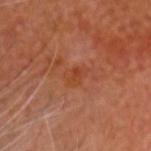follow-up = no biopsy performed (imaged during a skin exam); location = the head or neck; patient = male, in their mid-60s; acquisition = ~15 mm crop, total-body skin-cancer survey; lesion size = ≈2.5 mm; lighting = cross-polarized illumination.